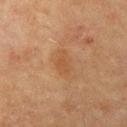- follow-up: imaged on a skin check; not biopsied
- imaging modality: ~15 mm crop, total-body skin-cancer survey
- body site: the left upper arm
- patient: female, in their 80s